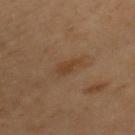Q: Was a biopsy performed?
A: imaged on a skin check; not biopsied
Q: Who is the patient?
A: female, approximately 60 years of age
Q: Lesion location?
A: the chest
Q: Illumination type?
A: cross-polarized illumination
Q: How was this image acquired?
A: ~15 mm tile from a whole-body skin photo